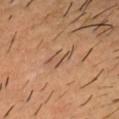{
  "biopsy_status": "not biopsied; imaged during a skin examination",
  "image": {
    "source": "total-body photography crop",
    "field_of_view_mm": 15
  },
  "lesion_size": {
    "long_diameter_mm_approx": 3.5
  },
  "site": "head or neck",
  "patient": {
    "sex": "male",
    "age_approx": 50
  }
}